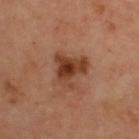notes: total-body-photography surveillance lesion; no biopsy | image-analysis metrics: a shape eccentricity near 0.5 and two-axis asymmetry of about 0.5; a border-irregularity rating of about 5.5/10, a color-variation rating of about 5.5/10, and peripheral color asymmetry of about 1.5; a lesion-detection confidence of about 100/100 | site: the back | imaging modality: ~15 mm crop, total-body skin-cancer survey | size: about 4 mm | subject: male, aged 63 to 67 | illumination: cross-polarized.